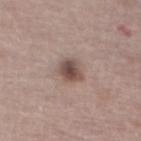Captured during whole-body skin photography for melanoma surveillance; the lesion was not biopsied. An algorithmic analysis of the crop reported a footprint of about 5.5 mm², an eccentricity of roughly 0.7, and a shape-asymmetry score of about 0.2 (0 = symmetric). The analysis additionally found a normalized lesion–skin contrast near 9.5. The analysis additionally found a border-irregularity index near 2/10, internal color variation of about 3.5 on a 0–10 scale, and a peripheral color-asymmetry measure near 1. A lesion tile, about 15 mm wide, cut from a 3D total-body photograph. The recorded lesion diameter is about 3 mm. This is a white-light tile. From the leg. A male patient approximately 65 years of age.